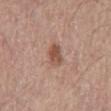Recorded during total-body skin imaging; not selected for excision or biopsy. From the mid back. A close-up tile cropped from a whole-body skin photograph, about 15 mm across. About 3 mm across. The lesion-visualizer software estimated an average lesion color of about L≈52 a*≈21 b*≈28 (CIELAB). Imaged with white-light lighting. A male subject, in their mid- to late 60s.